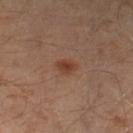Clinical impression: This lesion was catalogued during total-body skin photography and was not selected for biopsy. Context: The lesion's longest dimension is about 2.5 mm. A male patient, aged around 55. A close-up tile cropped from a whole-body skin photograph, about 15 mm across. Located on the left lower leg. This is a cross-polarized tile.